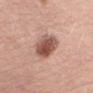| feature | finding |
|---|---|
| anatomic site | the right upper arm |
| image source | ~15 mm crop, total-body skin-cancer survey |
| subject | female, roughly 50 years of age |